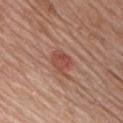This lesion was catalogued during total-body skin photography and was not selected for biopsy. A close-up tile cropped from a whole-body skin photograph, about 15 mm across. The patient is a male in their mid-70s. The tile uses white-light illumination. About 3 mm across. From the chest.